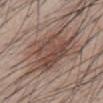Q: Is there a histopathology result?
A: catalogued during a skin exam; not biopsied
Q: What is the imaging modality?
A: total-body-photography crop, ~15 mm field of view
Q: Lesion size?
A: ≈7 mm
Q: Who is the patient?
A: male, in their 40s
Q: Illumination type?
A: white-light
Q: Automated lesion metrics?
A: a lesion area of about 24 mm² and an outline eccentricity of about 0.6 (0 = round, 1 = elongated); a border-irregularity index near 4.5/10, a within-lesion color-variation index near 6/10, and a peripheral color-asymmetry measure near 2
Q: Where on the body is the lesion?
A: the front of the torso